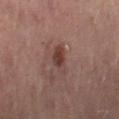Captured during whole-body skin photography for melanoma surveillance; the lesion was not biopsied.
A roughly 15 mm field-of-view crop from a total-body skin photograph.
The lesion is located on the left lower leg.
Captured under cross-polarized illumination.
A female patient aged around 55.